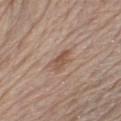<case>
  <biopsy_status>not biopsied; imaged during a skin examination</biopsy_status>
  <lesion_size>
    <long_diameter_mm_approx>3.0</long_diameter_mm_approx>
  </lesion_size>
  <image>
    <source>total-body photography crop</source>
    <field_of_view_mm>15</field_of_view_mm>
  </image>
  <site>chest</site>
  <patient>
    <sex>male</sex>
    <age_approx>80</age_approx>
  </patient>
</case>